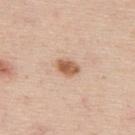This lesion was catalogued during total-body skin photography and was not selected for biopsy. The lesion is located on the upper back. The subject is a male aged 43 to 47. A 15 mm crop from a total-body photograph taken for skin-cancer surveillance.